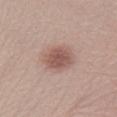Context:
Automated image analysis of the tile measured an area of roughly 10 mm² and an outline eccentricity of about 0.45 (0 = round, 1 = elongated). And it measured a nevus-likeness score of about 95/100. A female patient, aged 23 to 27. The lesion is on the right lower leg. A roughly 15 mm field-of-view crop from a total-body skin photograph.